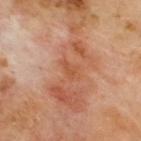biopsy_status: not biopsied; imaged during a skin examination
lighting: cross-polarized
patient:
  sex: male
  age_approx: 70
automated_metrics:
  border_irregularity_0_10: 3.0
  color_variation_0_10: 1.5
  lesion_detection_confidence_0_100: 100
image:
  source: total-body photography crop
  field_of_view_mm: 15
lesion_size:
  long_diameter_mm_approx: 3.0
site: upper back